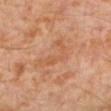Case summary:
– follow-up — total-body-photography surveillance lesion; no biopsy
– subject — male, in their 30s
– image — ~15 mm tile from a whole-body skin photo
– location — the left lower leg